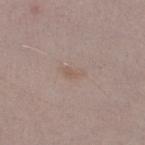Q: Was a biopsy performed?
A: catalogued during a skin exam; not biopsied
Q: What is the anatomic site?
A: the left thigh
Q: What kind of image is this?
A: 15 mm crop, total-body photography
Q: Patient demographics?
A: female, aged around 40